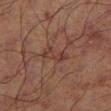Captured during whole-body skin photography for melanoma surveillance; the lesion was not biopsied. A region of skin cropped from a whole-body photographic capture, roughly 15 mm wide. The lesion is on the left lower leg. The patient is a male about 70 years old. About 3 mm across. Automated image analysis of the tile measured an area of roughly 4.5 mm², an eccentricity of roughly 0.85, and two-axis asymmetry of about 0.35. It also reported a detector confidence of about 100 out of 100 that the crop contains a lesion.